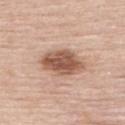Assessment:
Captured during whole-body skin photography for melanoma surveillance; the lesion was not biopsied.
Context:
About 5 mm across. This is a white-light tile. From the upper back. A male patient approximately 60 years of age. A 15 mm close-up extracted from a 3D total-body photography capture. Automated tile analysis of the lesion measured a footprint of about 13 mm², a shape eccentricity near 0.75, and a shape-asymmetry score of about 0.15 (0 = symmetric). The analysis additionally found a lesion color around L≈55 a*≈21 b*≈29 in CIELAB, a lesion–skin lightness drop of about 15, and a normalized border contrast of about 10. And it measured a border-irregularity index near 2/10, a within-lesion color-variation index near 5/10, and peripheral color asymmetry of about 1.5.